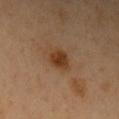The lesion was photographed on a routine skin check and not biopsied; there is no pathology result. A female patient, aged 48 to 52. Automated image analysis of the tile measured an eccentricity of roughly 0.35 and a symmetry-axis asymmetry near 0.2. The analysis additionally found a lesion–skin lightness drop of about 10. It also reported border irregularity of about 2 on a 0–10 scale and radial color variation of about 0.5. The recorded lesion diameter is about 2.5 mm. Cropped from a total-body skin-imaging series; the visible field is about 15 mm. This is a cross-polarized tile. On the left upper arm.